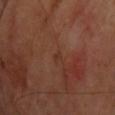biopsy_status: not biopsied; imaged during a skin examination
site: upper back
lesion_size:
  long_diameter_mm_approx: 1.0
automated_metrics:
  area_mm2_approx: 1.0
  eccentricity: 0.75
  shape_asymmetry: 0.4
  cielab_L: 32
  cielab_a: 20
  cielab_b: 27
  vs_skin_darker_L: 4.0
  color_variation_0_10: 0.0
  peripheral_color_asymmetry: 0.0
  nevus_likeness_0_100: 0
lighting: cross-polarized
image:
  source: total-body photography crop
  field_of_view_mm: 15
patient:
  sex: male
  age_approx: 65